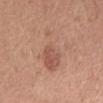Recorded during total-body skin imaging; not selected for excision or biopsy. A roughly 15 mm field-of-view crop from a total-body skin photograph. The subject is a male in their 70s. Longest diameter approximately 7.5 mm. On the chest. The tile uses white-light illumination.